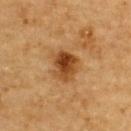Clinical impression:
Captured during whole-body skin photography for melanoma surveillance; the lesion was not biopsied.
Image and clinical context:
The total-body-photography lesion software estimated an average lesion color of about L≈39 a*≈21 b*≈36 (CIELAB), roughly 12 lightness units darker than nearby skin, and a normalized border contrast of about 10. A male subject, aged 83 or over. A region of skin cropped from a whole-body photographic capture, roughly 15 mm wide. The lesion is located on the upper back. The recorded lesion diameter is about 3.5 mm.